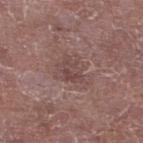{
  "lesion_size": {
    "long_diameter_mm_approx": 3.0
  },
  "patient": {
    "sex": "male",
    "age_approx": 65
  },
  "image": {
    "source": "total-body photography crop",
    "field_of_view_mm": 15
  },
  "automated_metrics": {
    "border_irregularity_0_10": 7.0,
    "color_variation_0_10": 0.5,
    "peripheral_color_asymmetry": 0.0
  },
  "site": "right lower leg",
  "lighting": "white-light"
}